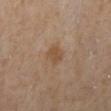Q: Was a biopsy performed?
A: imaged on a skin check; not biopsied